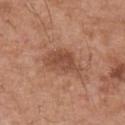The lesion was tiled from a total-body skin photograph and was not biopsied. An algorithmic analysis of the crop reported an area of roughly 11 mm² and two-axis asymmetry of about 0.35. It also reported border irregularity of about 4 on a 0–10 scale, a color-variation rating of about 2.5/10, and peripheral color asymmetry of about 1. The analysis additionally found a lesion-detection confidence of about 100/100. Imaged with white-light lighting. The patient is a male aged 53–57. A 15 mm close-up tile from a total-body photography series done for melanoma screening. The lesion is on the right upper arm.